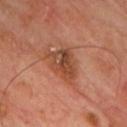<record>
<biopsy_status>not biopsied; imaged during a skin examination</biopsy_status>
<lighting>cross-polarized</lighting>
<image>
  <source>total-body photography crop</source>
  <field_of_view_mm>15</field_of_view_mm>
</image>
<site>chest</site>
<patient>
  <sex>male</sex>
  <age_approx>65</age_approx>
</patient>
<lesion_size>
  <long_diameter_mm_approx>4.0</long_diameter_mm_approx>
</lesion_size>
<automated_metrics>
  <area_mm2_approx>10.0</area_mm2_approx>
  <eccentricity>0.65</eccentricity>
  <border_irregularity_0_10>3.5</border_irregularity_0_10>
  <color_variation_0_10>6.5</color_variation_0_10>
  <peripheral_color_asymmetry>2.0</peripheral_color_asymmetry>
  <nevus_likeness_0_100>5</nevus_likeness_0_100>
  <lesion_detection_confidence_0_100>100</lesion_detection_confidence_0_100>
</automated_metrics>
</record>